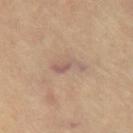Part of a total-body skin-imaging series; this lesion was reviewed on a skin check and was not flagged for biopsy. A 15 mm crop from a total-body photograph taken for skin-cancer surveillance. The lesion is on the chest. About 4 mm across. Imaged with cross-polarized lighting. The lesion-visualizer software estimated a lesion area of about 4 mm² and an eccentricity of roughly 0.9. And it measured a lesion color around L≈58 a*≈17 b*≈25 in CIELAB, about 8 CIELAB-L* units darker than the surrounding skin, and a normalized lesion–skin contrast near 6. The analysis additionally found a border-irregularity index near 6.5/10 and peripheral color asymmetry of about 0.5.